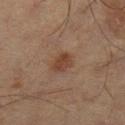Recorded during total-body skin imaging; not selected for excision or biopsy. A 15 mm close-up extracted from a 3D total-body photography capture. A male patient about 60 years old. The lesion is located on the leg. The lesion's longest dimension is about 3 mm.